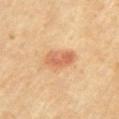Case summary:
– workup: imaged on a skin check; not biopsied
– image-analysis metrics: a lesion area of about 8 mm², an eccentricity of roughly 0.85, and two-axis asymmetry of about 0.2; an average lesion color of about L≈60 a*≈24 b*≈36 (CIELAB) and roughly 10 lightness units darker than nearby skin; a color-variation rating of about 4.5/10 and a peripheral color-asymmetry measure near 2; a nevus-likeness score of about 95/100 and a detector confidence of about 100 out of 100 that the crop contains a lesion
– size: ~4 mm (longest diameter)
– anatomic site: the chest
– subject: male, roughly 70 years of age
– imaging modality: 15 mm crop, total-body photography
– illumination: cross-polarized